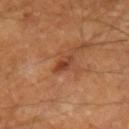Clinical impression:
Imaged during a routine full-body skin examination; the lesion was not biopsied and no histopathology is available.
Image and clinical context:
The lesion's longest dimension is about 2.5 mm. The lesion is located on the right forearm. A 15 mm crop from a total-body photograph taken for skin-cancer surveillance. Automated image analysis of the tile measured border irregularity of about 3 on a 0–10 scale and peripheral color asymmetry of about 1. A male subject aged approximately 85 or older.